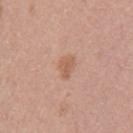Imaged during a routine full-body skin examination; the lesion was not biopsied and no histopathology is available.
The lesion's longest dimension is about 2.5 mm.
The lesion-visualizer software estimated border irregularity of about 2.5 on a 0–10 scale and a color-variation rating of about 1.5/10. It also reported an automated nevus-likeness rating near 45 out of 100 and lesion-presence confidence of about 100/100.
The tile uses white-light illumination.
A female subject aged 33 to 37.
A region of skin cropped from a whole-body photographic capture, roughly 15 mm wide.
From the left upper arm.